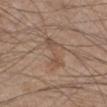Q: Is there a histopathology result?
A: imaged on a skin check; not biopsied